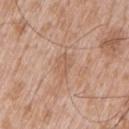No biopsy was performed on this lesion — it was imaged during a full skin examination and was not determined to be concerning. The lesion is located on the left upper arm. The lesion-visualizer software estimated a lesion color around L≈58 a*≈20 b*≈31 in CIELAB and a lesion–skin lightness drop of about 7. The analysis additionally found a classifier nevus-likeness of about 0/100. The lesion's longest dimension is about 2.5 mm. A 15 mm crop from a total-body photograph taken for skin-cancer surveillance. A male subject, in their 50s.